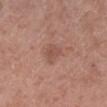Notes:
* workup · catalogued during a skin exam; not biopsied
* image source · 15 mm crop, total-body photography
* illumination · white-light illumination
* anatomic site · the right lower leg
* patient · male, in their 70s
* lesion diameter · ~2.5 mm (longest diameter)